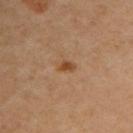Case summary:
- workup · catalogued during a skin exam; not biopsied
- body site · the arm
- diameter · ≈2 mm
- acquisition · ~15 mm tile from a whole-body skin photo
- lighting · cross-polarized illumination
- patient · female, aged 43–47